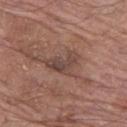Imaged during a routine full-body skin examination; the lesion was not biopsied and no histopathology is available. A 15 mm crop from a total-body photograph taken for skin-cancer surveillance. About 4 mm across. A male subject, in their mid- to late 70s. On the left thigh. Imaged with white-light lighting.